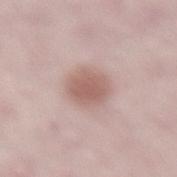{
  "biopsy_status": "not biopsied; imaged during a skin examination",
  "patient": {
    "sex": "female",
    "age_approx": 40
  },
  "automated_metrics": {
    "area_mm2_approx": 10.0,
    "eccentricity": 0.45,
    "shape_asymmetry": 0.15,
    "cielab_L": 59,
    "cielab_a": 19,
    "cielab_b": 23,
    "vs_skin_contrast_norm": 7.5,
    "nevus_likeness_0_100": 80,
    "lesion_detection_confidence_0_100": 100
  },
  "lighting": "white-light",
  "site": "lower back",
  "lesion_size": {
    "long_diameter_mm_approx": 4.0
  },
  "image": {
    "source": "total-body photography crop",
    "field_of_view_mm": 15
  }
}